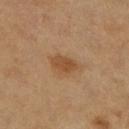Assessment: The lesion was photographed on a routine skin check and not biopsied; there is no pathology result. Background: A 15 mm close-up tile from a total-body photography series done for melanoma screening. From the right lower leg. A female patient approximately 60 years of age. Captured under cross-polarized illumination.